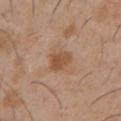No biopsy was performed on this lesion — it was imaged during a full skin examination and was not determined to be concerning.
A roughly 15 mm field-of-view crop from a total-body skin photograph.
The patient is a male roughly 30 years of age.
From the chest.
The lesion-visualizer software estimated an outline eccentricity of about 0.65 (0 = round, 1 = elongated) and two-axis asymmetry of about 0.2. The software also gave an average lesion color of about L≈51 a*≈20 b*≈33 (CIELAB), a lesion–skin lightness drop of about 9, and a normalized lesion–skin contrast near 7.5. It also reported border irregularity of about 2 on a 0–10 scale and a color-variation rating of about 3/10.
Imaged with white-light lighting.
Approximately 2.5 mm at its widest.